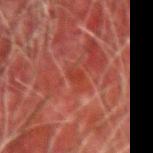On the right forearm.
Captured under cross-polarized illumination.
A 15 mm close-up tile from a total-body photography series done for melanoma screening.
A male subject aged around 80.
The recorded lesion diameter is about 3 mm.
Automated tile analysis of the lesion measured a lesion–skin lightness drop of about 5 and a normalized border contrast of about 6. The software also gave a classifier nevus-likeness of about 0/100 and a lesion-detection confidence of about 60/100.